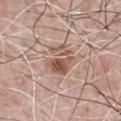A 15 mm crop from a total-body photograph taken for skin-cancer surveillance.
A male patient, about 65 years old.
Approximately 4 mm at its widest.
Captured under white-light illumination.
The lesion is on the chest.
The lesion-visualizer software estimated a lesion area of about 9 mm² and an eccentricity of roughly 0.6. The analysis additionally found a within-lesion color-variation index near 5.5/10 and peripheral color asymmetry of about 2.